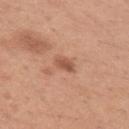Captured under white-light illumination. Located on the right upper arm. A 15 mm close-up extracted from a 3D total-body photography capture. The subject is a female aged 28 to 32. The total-body-photography lesion software estimated an average lesion color of about L≈54 a*≈23 b*≈32 (CIELAB), a lesion–skin lightness drop of about 10, and a normalized lesion–skin contrast near 7. It also reported border irregularity of about 3 on a 0–10 scale, internal color variation of about 2 on a 0–10 scale, and a peripheral color-asymmetry measure near 1. Measured at roughly 2.5 mm in maximum diameter.